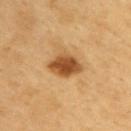workup: no biopsy performed (imaged during a skin exam) | tile lighting: cross-polarized | image-analysis metrics: a footprint of about 8.5 mm² and two-axis asymmetry of about 0.2; a border-irregularity rating of about 2/10, a within-lesion color-variation index near 4.5/10, and peripheral color asymmetry of about 1.5 | subject: male, in their 60s | imaging modality: 15 mm crop, total-body photography | diameter: ~4 mm (longest diameter) | body site: the upper back.